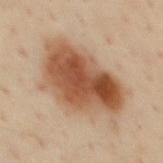Assessment: Recorded during total-body skin imaging; not selected for excision or biopsy. Acquisition and patient details: The lesion is on the mid back. A male subject, aged around 45. A region of skin cropped from a whole-body photographic capture, roughly 15 mm wide. About 9.5 mm across. Automated image analysis of the tile measured a lesion area of about 42 mm² and a shape eccentricity near 0.75. The software also gave a mean CIELAB color near L≈53 a*≈20 b*≈32 and a normalized border contrast of about 10. The software also gave a nevus-likeness score of about 100/100 and a detector confidence of about 100 out of 100 that the crop contains a lesion. Captured under cross-polarized illumination.